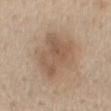biopsy status: imaged on a skin check; not biopsied
patient: male, roughly 65 years of age
size: about 6.5 mm
location: the mid back
imaging modality: total-body-photography crop, ~15 mm field of view
tile lighting: white-light